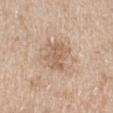Captured during whole-body skin photography for melanoma surveillance; the lesion was not biopsied.
A region of skin cropped from a whole-body photographic capture, roughly 15 mm wide.
The lesion's longest dimension is about 3.5 mm.
A female subject, aged 68 to 72.
On the right lower leg.
Imaged with white-light lighting.